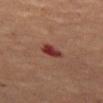Q: Is there a histopathology result?
A: no biopsy performed (imaged during a skin exam)
Q: Lesion location?
A: the left thigh
Q: How was this image acquired?
A: ~15 mm crop, total-body skin-cancer survey
Q: What did automated image analysis measure?
A: a lesion area of about 4 mm², a shape eccentricity near 0.8, and two-axis asymmetry of about 0.25; a border-irregularity rating of about 2/10, a color-variation rating of about 4.5/10, and a peripheral color-asymmetry measure near 1.5
Q: What is the lesion's diameter?
A: about 3 mm
Q: What are the patient's age and sex?
A: female, aged around 60
Q: Illumination type?
A: cross-polarized